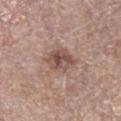Recorded during total-body skin imaging; not selected for excision or biopsy.
The tile uses white-light illumination.
On the leg.
A female patient, approximately 70 years of age.
Measured at roughly 4 mm in maximum diameter.
A 15 mm crop from a total-body photograph taken for skin-cancer surveillance.